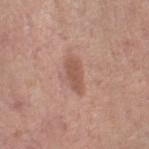| key | value |
|---|---|
| follow-up | imaged on a skin check; not biopsied |
| anatomic site | the leg |
| subject | male, aged approximately 75 |
| illumination | white-light |
| image | 15 mm crop, total-body photography |